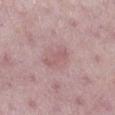Clinical impression:
The lesion was photographed on a routine skin check and not biopsied; there is no pathology result.
Context:
A 15 mm close-up tile from a total-body photography series done for melanoma screening. The recorded lesion diameter is about 3.5 mm. This is a white-light tile. The lesion-visualizer software estimated an average lesion color of about L≈57 a*≈22 b*≈19 (CIELAB), roughly 7 lightness units darker than nearby skin, and a lesion-to-skin contrast of about 5 (normalized; higher = more distinct). The software also gave a within-lesion color-variation index near 0.5/10. The software also gave a classifier nevus-likeness of about 0/100 and a detector confidence of about 100 out of 100 that the crop contains a lesion. The patient is a female aged approximately 45. The lesion is located on the right lower leg.